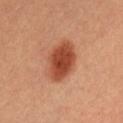Part of a total-body skin-imaging series; this lesion was reviewed on a skin check and was not flagged for biopsy. A female subject, aged around 30. Automated image analysis of the tile measured a footprint of about 14 mm², an outline eccentricity of about 0.75 (0 = round, 1 = elongated), and a shape-asymmetry score of about 0.1 (0 = symmetric). It also reported a border-irregularity rating of about 1.5/10, a within-lesion color-variation index near 3.5/10, and peripheral color asymmetry of about 1. The software also gave an automated nevus-likeness rating near 100 out of 100 and a detector confidence of about 100 out of 100 that the crop contains a lesion. Located on the abdomen. The recorded lesion diameter is about 5.5 mm. A region of skin cropped from a whole-body photographic capture, roughly 15 mm wide.